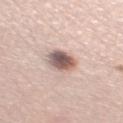  biopsy_status: not biopsied; imaged during a skin examination
  lesion_size:
    long_diameter_mm_approx: 3.5
  site: left thigh
  lighting: white-light
  image:
    source: total-body photography crop
    field_of_view_mm: 15
  patient:
    sex: male
    age_approx: 65